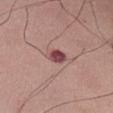| feature | finding |
|---|---|
| workup | no biopsy performed (imaged during a skin exam) |
| tile lighting | white-light |
| image-analysis metrics | an eccentricity of roughly 0.65 and two-axis asymmetry of about 0.15; a border-irregularity index near 1.5/10, a color-variation rating of about 4/10, and peripheral color asymmetry of about 1.5 |
| location | the right upper arm |
| image | ~15 mm crop, total-body skin-cancer survey |
| size | ~2.5 mm (longest diameter) |
| subject | male, approximately 50 years of age |